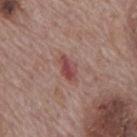{"biopsy_status": "not biopsied; imaged during a skin examination", "image": {"source": "total-body photography crop", "field_of_view_mm": 15}, "patient": {"sex": "male", "age_approx": 70}, "site": "back", "lesion_size": {"long_diameter_mm_approx": 3.0}}